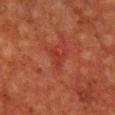The lesion was tiled from a total-body skin photograph and was not biopsied. A roughly 15 mm field-of-view crop from a total-body skin photograph. Imaged with cross-polarized lighting. Measured at roughly 2.5 mm in maximum diameter. The lesion is located on the chest. An algorithmic analysis of the crop reported a lesion area of about 3 mm² and an outline eccentricity of about 0.85 (0 = round, 1 = elongated). And it measured roughly 5 lightness units darker than nearby skin. The software also gave an automated nevus-likeness rating near 0 out of 100 and lesion-presence confidence of about 90/100. A male patient roughly 60 years of age.